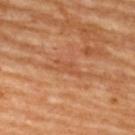Automated image analysis of the tile measured a shape-asymmetry score of about 0.4 (0 = symmetric). The analysis additionally found a lesion–skin lightness drop of about 6 and a lesion-to-skin contrast of about 4.5 (normalized; higher = more distinct). And it measured a color-variation rating of about 0/10 and peripheral color asymmetry of about 0. The software also gave a detector confidence of about 65 out of 100 that the crop contains a lesion.
On the upper back.
This is a cross-polarized tile.
About 3.5 mm across.
A 15 mm crop from a total-body photograph taken for skin-cancer surveillance.
A female patient roughly 65 years of age.